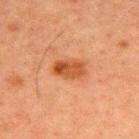| field | value |
|---|---|
| biopsy status | total-body-photography surveillance lesion; no biopsy |
| body site | the upper back |
| tile lighting | cross-polarized |
| image | 15 mm crop, total-body photography |
| lesion diameter | ≈4 mm |
| subject | male, in their 60s |